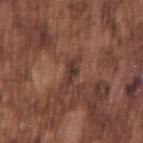biopsy status = catalogued during a skin exam; not biopsied.